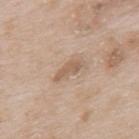– notes: no biopsy performed (imaged during a skin exam)
– imaging modality: ~15 mm crop, total-body skin-cancer survey
– anatomic site: the upper back
– patient: male, aged 63–67